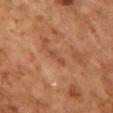Notes:
– follow-up · imaged on a skin check; not biopsied
– imaging modality · ~15 mm crop, total-body skin-cancer survey
– illumination · cross-polarized illumination
– image-analysis metrics · a mean CIELAB color near L≈42 a*≈22 b*≈31 and a lesion-to-skin contrast of about 5 (normalized; higher = more distinct); a nevus-likeness score of about 0/100 and a detector confidence of about 80 out of 100 that the crop contains a lesion
– body site · the front of the torso
– subject · female, about 60 years old
– size · ~2.5 mm (longest diameter)